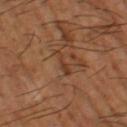Assessment: Part of a total-body skin-imaging series; this lesion was reviewed on a skin check and was not flagged for biopsy. Background: A male patient aged 63 to 67. From the left thigh. A close-up tile cropped from a whole-body skin photograph, about 15 mm across. The lesion-visualizer software estimated a lesion area of about 3.5 mm² and a shape-asymmetry score of about 0.4 (0 = symmetric). The software also gave a lesion color around L≈39 a*≈21 b*≈31 in CIELAB, about 7 CIELAB-L* units darker than the surrounding skin, and a lesion-to-skin contrast of about 6 (normalized; higher = more distinct). The software also gave border irregularity of about 5 on a 0–10 scale, a color-variation rating of about 0.5/10, and peripheral color asymmetry of about 0. Captured under cross-polarized illumination. The recorded lesion diameter is about 3 mm.